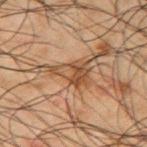{
  "biopsy_status": "not biopsied; imaged during a skin examination",
  "image": {
    "source": "total-body photography crop",
    "field_of_view_mm": 15
  },
  "site": "right upper arm",
  "patient": {
    "sex": "male",
    "age_approx": 50
  },
  "lesion_size": {
    "long_diameter_mm_approx": 4.0
  },
  "automated_metrics": {
    "shape_asymmetry": 0.45,
    "cielab_L": 37,
    "cielab_a": 16,
    "cielab_b": 27,
    "vs_skin_darker_L": 8.0,
    "vs_skin_contrast_norm": 7.0,
    "border_irregularity_0_10": 4.5,
    "color_variation_0_10": 6.5,
    "peripheral_color_asymmetry": 2.5,
    "nevus_likeness_0_100": 0,
    "lesion_detection_confidence_0_100": 80
  },
  "lighting": "cross-polarized"
}